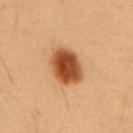Assessment: The lesion was photographed on a routine skin check and not biopsied; there is no pathology result. Acquisition and patient details: The tile uses cross-polarized illumination. A roughly 15 mm field-of-view crop from a total-body skin photograph. The lesion is located on the front of the torso. Automated image analysis of the tile measured a mean CIELAB color near L≈49 a*≈25 b*≈39, a lesion–skin lightness drop of about 18, and a lesion-to-skin contrast of about 12 (normalized; higher = more distinct). It also reported a lesion-detection confidence of about 100/100. A male patient, about 55 years old. Measured at roughly 4.5 mm in maximum diameter.